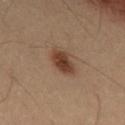Findings:
- biopsy status · total-body-photography surveillance lesion; no biopsy
- patient · male, roughly 55 years of age
- anatomic site · the abdomen
- acquisition · ~15 mm crop, total-body skin-cancer survey